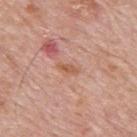Clinical impression:
The lesion was tiled from a total-body skin photograph and was not biopsied.
Acquisition and patient details:
About 2.5 mm across. A male patient, approximately 80 years of age. This is a white-light tile. A 15 mm crop from a total-body photograph taken for skin-cancer surveillance. On the upper back.